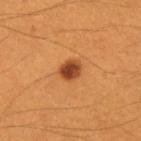Case summary:
* notes: imaged on a skin check; not biopsied
* TBP lesion metrics: a footprint of about 5 mm², an eccentricity of roughly 0.45, and a shape-asymmetry score of about 0.15 (0 = symmetric)
* subject: female, aged 38–42
* imaging modality: ~15 mm crop, total-body skin-cancer survey
* tile lighting: cross-polarized illumination
* diameter: ≈2.5 mm
* site: the left forearm